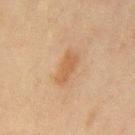biopsy_status: not biopsied; imaged during a skin examination
image:
  source: total-body photography crop
  field_of_view_mm: 15
lesion_size:
  long_diameter_mm_approx: 4.0
automated_metrics:
  cielab_L: 48
  cielab_a: 17
  cielab_b: 31
  vs_skin_darker_L: 7.0
  vs_skin_contrast_norm: 6.5
  border_irregularity_0_10: 2.5
  color_variation_0_10: 1.5
  peripheral_color_asymmetry: 0.5
site: mid back
lighting: cross-polarized
patient:
  sex: male
  age_approx: 45